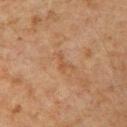Assessment: The lesion was tiled from a total-body skin photograph and was not biopsied. Clinical summary: The lesion is on the right upper arm. The tile uses cross-polarized illumination. The patient is a male approximately 60 years of age. A 15 mm crop from a total-body photograph taken for skin-cancer surveillance.